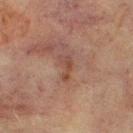Imaged during a routine full-body skin examination; the lesion was not biopsied and no histopathology is available.
Imaged with cross-polarized lighting.
The patient is a male in their 70s.
Measured at roughly 3 mm in maximum diameter.
From the chest.
A 15 mm crop from a total-body photograph taken for skin-cancer surveillance.